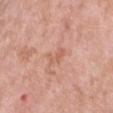Assessment:
Recorded during total-body skin imaging; not selected for excision or biopsy.
Background:
A close-up tile cropped from a whole-body skin photograph, about 15 mm across. On the chest. The subject is a male aged approximately 50.